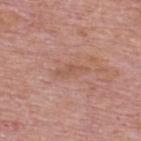follow-up — no biopsy performed (imaged during a skin exam) | patient — male, aged 73–77 | location — the upper back | lesion diameter — ≈3.5 mm | lighting — white-light | image source — ~15 mm tile from a whole-body skin photo | automated metrics — an area of roughly 3 mm², an eccentricity of roughly 0.95, and a symmetry-axis asymmetry near 0.4; an average lesion color of about L≈54 a*≈22 b*≈29 (CIELAB) and roughly 7 lightness units darker than nearby skin; a border-irregularity rating of about 5.5/10, internal color variation of about 0 on a 0–10 scale, and peripheral color asymmetry of about 0; a nevus-likeness score of about 0/100 and lesion-presence confidence of about 95/100.